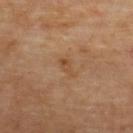Part of a total-body skin-imaging series; this lesion was reviewed on a skin check and was not flagged for biopsy. An algorithmic analysis of the crop reported border irregularity of about 4.5 on a 0–10 scale, internal color variation of about 0 on a 0–10 scale, and peripheral color asymmetry of about 0. A 15 mm crop from a total-body photograph taken for skin-cancer surveillance. A male subject roughly 70 years of age. Imaged with cross-polarized lighting. On the upper back.